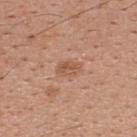No biopsy was performed on this lesion — it was imaged during a full skin examination and was not determined to be concerning.
A male subject, aged around 30.
A 15 mm close-up tile from a total-body photography series done for melanoma screening.
The lesion is located on the upper back.